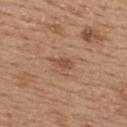– notes: total-body-photography surveillance lesion; no biopsy
– patient: female, aged 33–37
– body site: the upper back
– automated lesion analysis: a lesion area of about 3 mm², an outline eccentricity of about 0.8 (0 = round, 1 = elongated), and a symmetry-axis asymmetry near 0.35; about 8 CIELAB-L* units darker than the surrounding skin and a lesion-to-skin contrast of about 6 (normalized; higher = more distinct)
– acquisition: total-body-photography crop, ~15 mm field of view
– illumination: white-light